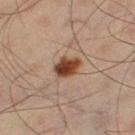The lesion was tiled from a total-body skin photograph and was not biopsied. Captured under cross-polarized illumination. Located on the right thigh. A lesion tile, about 15 mm wide, cut from a 3D total-body photograph. The patient is a male about 60 years old.